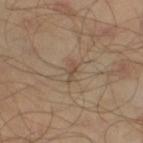Impression: The lesion was tiled from a total-body skin photograph and was not biopsied. Clinical summary: Captured under cross-polarized illumination. The recorded lesion diameter is about 2.5 mm. The lesion-visualizer software estimated a footprint of about 2.5 mm² and an eccentricity of roughly 0.9. And it measured an automated nevus-likeness rating near 0 out of 100 and a lesion-detection confidence of about 95/100. The patient is a male in their mid- to late 40s. A 15 mm close-up tile from a total-body photography series done for melanoma screening. On the right thigh.